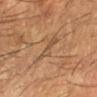Part of a total-body skin-imaging series; this lesion was reviewed on a skin check and was not flagged for biopsy. This is a cross-polarized tile. A 15 mm close-up tile from a total-body photography series done for melanoma screening. The lesion is located on the left forearm. A male subject, aged approximately 65.